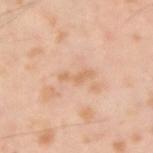<tbp_lesion>
<biopsy_status>not biopsied; imaged during a skin examination</biopsy_status>
<image>
  <source>total-body photography crop</source>
  <field_of_view_mm>15</field_of_view_mm>
</image>
<lesion_size>
  <long_diameter_mm_approx>3.5</long_diameter_mm_approx>
</lesion_size>
<lighting>cross-polarized</lighting>
<site>right upper arm</site>
<patient>
  <sex>male</sex>
  <age_approx>55</age_approx>
</patient>
</tbp_lesion>